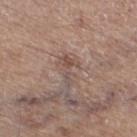Assessment:
Recorded during total-body skin imaging; not selected for excision or biopsy.
Image and clinical context:
A region of skin cropped from a whole-body photographic capture, roughly 15 mm wide. On the right thigh. A male subject, in their mid- to late 60s. The tile uses white-light illumination. Automated image analysis of the tile measured a footprint of about 4.5 mm² and an eccentricity of roughly 0.9. And it measured a lesion–skin lightness drop of about 7 and a lesion-to-skin contrast of about 5.5 (normalized; higher = more distinct). It also reported a classifier nevus-likeness of about 0/100 and a lesion-detection confidence of about 75/100. The recorded lesion diameter is about 4 mm.